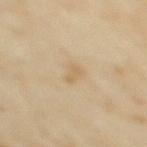Impression:
Recorded during total-body skin imaging; not selected for excision or biopsy.
Image and clinical context:
The patient is a female aged 33–37. The lesion-visualizer software estimated a lesion area of about 4 mm², an outline eccentricity of about 0.8 (0 = round, 1 = elongated), and a symmetry-axis asymmetry near 0.25. It also reported border irregularity of about 2.5 on a 0–10 scale and a color-variation rating of about 1.5/10. The lesion's longest dimension is about 3 mm. The lesion is on the back. This image is a 15 mm lesion crop taken from a total-body photograph. This is a cross-polarized tile.